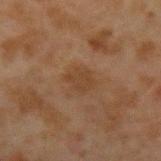This lesion was catalogued during total-body skin photography and was not selected for biopsy.
Imaged with cross-polarized lighting.
Automated image analysis of the tile measured an average lesion color of about L≈33 a*≈15 b*≈27 (CIELAB) and about 5 CIELAB-L* units darker than the surrounding skin. And it measured a nevus-likeness score of about 0/100 and a lesion-detection confidence of about 100/100.
Located on the left forearm.
About 3 mm across.
A region of skin cropped from a whole-body photographic capture, roughly 15 mm wide.
A male subject, about 45 years old.